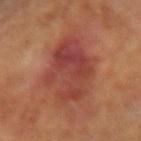Cropped from a whole-body photographic skin survey; the tile spans about 15 mm. Longest diameter approximately 7.5 mm. From the left arm. A female patient roughly 65 years of age.